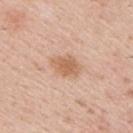Case summary:
* notes — no biopsy performed (imaged during a skin exam)
* TBP lesion metrics — a border-irregularity index near 1.5/10 and a within-lesion color-variation index near 2.5/10; lesion-presence confidence of about 100/100
* acquisition — total-body-photography crop, ~15 mm field of view
* location — the right upper arm
* subject — male, about 40 years old
* size — ~3.5 mm (longest diameter)
* illumination — white-light